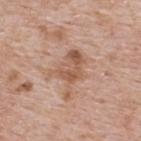workup=catalogued during a skin exam; not biopsied | illumination=white-light illumination | site=the upper back | lesion size=≈5.5 mm | acquisition=total-body-photography crop, ~15 mm field of view | subject=male, aged 63–67.